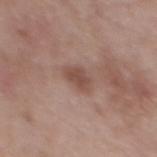Clinical impression: The lesion was tiled from a total-body skin photograph and was not biopsied. Image and clinical context: The tile uses white-light illumination. The lesion-visualizer software estimated a lesion area of about 4.5 mm² and a symmetry-axis asymmetry near 0.3. The software also gave an automated nevus-likeness rating near 5 out of 100. A roughly 15 mm field-of-view crop from a total-body skin photograph. Measured at roughly 3 mm in maximum diameter. The patient is a male aged 53–57. The lesion is located on the mid back.